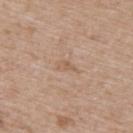{
  "biopsy_status": "not biopsied; imaged during a skin examination",
  "lesion_size": {
    "long_diameter_mm_approx": 2.5
  },
  "image": {
    "source": "total-body photography crop",
    "field_of_view_mm": 15
  },
  "automated_metrics": {
    "area_mm2_approx": 2.5,
    "eccentricity": 0.9,
    "shape_asymmetry": 0.4,
    "vs_skin_contrast_norm": 4.5,
    "border_irregularity_0_10": 3.5,
    "peripheral_color_asymmetry": 0.0,
    "nevus_likeness_0_100": 0
  },
  "lighting": "white-light",
  "site": "upper back",
  "patient": {
    "sex": "female",
    "age_approx": 40
  }
}